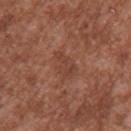The lesion was tiled from a total-body skin photograph and was not biopsied.
The patient is a male aged approximately 45.
Automated tile analysis of the lesion measured an area of roughly 5.5 mm², a shape eccentricity near 0.8, and a shape-asymmetry score of about 0.35 (0 = symmetric). And it measured a mean CIELAB color near L≈42 a*≈23 b*≈28 and a normalized border contrast of about 5.
A 15 mm close-up extracted from a 3D total-body photography capture.
The lesion is located on the upper back.
Captured under white-light illumination.
About 3.5 mm across.